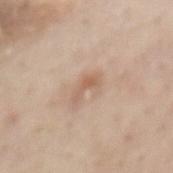<case>
  <site>mid back</site>
  <image>
    <source>total-body photography crop</source>
    <field_of_view_mm>15</field_of_view_mm>
  </image>
  <patient>
    <sex>male</sex>
    <age_approx>55</age_approx>
  </patient>
</case>